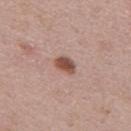| key | value |
|---|---|
| workup | imaged on a skin check; not biopsied |
| tile lighting | white-light illumination |
| body site | the mid back |
| patient | male, roughly 45 years of age |
| lesion size | ≈2.5 mm |
| acquisition | ~15 mm crop, total-body skin-cancer survey |
| automated metrics | an area of roughly 4.5 mm², an outline eccentricity of about 0.7 (0 = round, 1 = elongated), and a shape-asymmetry score of about 0.2 (0 = symmetric); a mean CIELAB color near L≈51 a*≈22 b*≈26, a lesion–skin lightness drop of about 14, and a lesion-to-skin contrast of about 9.5 (normalized; higher = more distinct); a border-irregularity index near 2/10, a within-lesion color-variation index near 2.5/10, and a peripheral color-asymmetry measure near 1; a classifier nevus-likeness of about 100/100 and a lesion-detection confidence of about 100/100 |